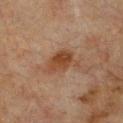  biopsy_status: not biopsied; imaged during a skin examination
  lighting: cross-polarized
  image:
    source: total-body photography crop
    field_of_view_mm: 15
  patient:
    sex: female
    age_approx: 55
  site: chest